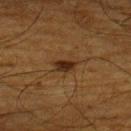The lesion was tiled from a total-body skin photograph and was not biopsied.
A male patient, aged approximately 65.
From the back.
An algorithmic analysis of the crop reported an outline eccentricity of about 0.8 (0 = round, 1 = elongated) and a shape-asymmetry score of about 0.25 (0 = symmetric).
A close-up tile cropped from a whole-body skin photograph, about 15 mm across.
Imaged with cross-polarized lighting.
Longest diameter approximately 2.5 mm.